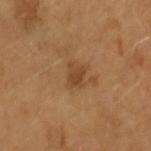follow-up — total-body-photography surveillance lesion; no biopsy | body site — the left forearm | acquisition — 15 mm crop, total-body photography | size — about 2.5 mm | patient — female, aged approximately 55 | tile lighting — cross-polarized illumination.